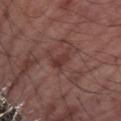This lesion was catalogued during total-body skin photography and was not selected for biopsy. The lesion is on the left forearm. Cropped from a total-body skin-imaging series; the visible field is about 15 mm. The recorded lesion diameter is about 3 mm. The subject is a male aged around 70. The tile uses white-light illumination.